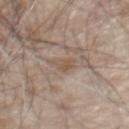Imaged during a routine full-body skin examination; the lesion was not biopsied and no histopathology is available.
A male subject approximately 80 years of age.
The tile uses white-light illumination.
The lesion is on the chest.
A region of skin cropped from a whole-body photographic capture, roughly 15 mm wide.
An algorithmic analysis of the crop reported a lesion area of about 3.5 mm², an outline eccentricity of about 0.7 (0 = round, 1 = elongated), and a symmetry-axis asymmetry near 0.3. The software also gave a border-irregularity rating of about 3/10 and a peripheral color-asymmetry measure near 1.
Approximately 2.5 mm at its widest.